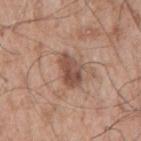  biopsy_status: not biopsied; imaged during a skin examination
  automated_metrics:
    area_mm2_approx: 7.5
    shape_asymmetry: 0.2
    border_irregularity_0_10: 2.5
    color_variation_0_10: 4.0
    nevus_likeness_0_100: 15
    lesion_detection_confidence_0_100: 100
  lesion_size:
    long_diameter_mm_approx: 4.0
  lighting: white-light
  site: mid back
  patient:
    sex: male
    age_approx: 55
  image:
    source: total-body photography crop
    field_of_view_mm: 15